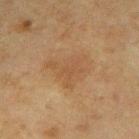{"biopsy_status": "not biopsied; imaged during a skin examination", "image": {"source": "total-body photography crop", "field_of_view_mm": 15}, "site": "left upper arm", "lesion_size": {"long_diameter_mm_approx": 5.0}, "lighting": "cross-polarized", "patient": {"sex": "male", "age_approx": 65}}